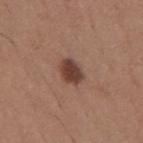This lesion was catalogued during total-body skin photography and was not selected for biopsy. A roughly 15 mm field-of-view crop from a total-body skin photograph. The lesion is on the mid back. Approximately 3.5 mm at its widest. A male patient about 65 years old. Imaged with white-light lighting.